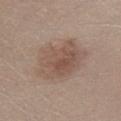{
  "biopsy_status": "not biopsied; imaged during a skin examination",
  "site": "right lower leg",
  "patient": {
    "sex": "female",
    "age_approx": 55
  },
  "image": {
    "source": "total-body photography crop",
    "field_of_view_mm": 15
  }
}